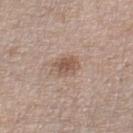Part of a total-body skin-imaging series; this lesion was reviewed on a skin check and was not flagged for biopsy.
Located on the right thigh.
The patient is a female aged 58–62.
Automated tile analysis of the lesion measured a lesion color around L≈54 a*≈17 b*≈26 in CIELAB and a lesion-to-skin contrast of about 7 (normalized; higher = more distinct).
A close-up tile cropped from a whole-body skin photograph, about 15 mm across.
About 3 mm across.